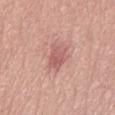• biopsy status · catalogued during a skin exam; not biopsied
• diameter · about 3.5 mm
• TBP lesion metrics · an area of roughly 6 mm², an eccentricity of roughly 0.85, and two-axis asymmetry of about 0.2; a mean CIELAB color near L≈58 a*≈26 b*≈24 and a normalized border contrast of about 6.5; internal color variation of about 3.5 on a 0–10 scale and radial color variation of about 1.5
• subject · male, aged 68–72
• anatomic site · the left thigh
• imaging modality · total-body-photography crop, ~15 mm field of view